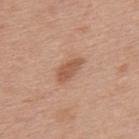The lesion was photographed on a routine skin check and not biopsied; there is no pathology result.
A region of skin cropped from a whole-body photographic capture, roughly 15 mm wide.
The lesion's longest dimension is about 3.5 mm.
Automated tile analysis of the lesion measured border irregularity of about 2.5 on a 0–10 scale, a within-lesion color-variation index near 2/10, and a peripheral color-asymmetry measure near 0.5.
The lesion is located on the upper back.
A male patient, roughly 30 years of age.